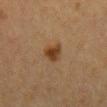Findings:
– biopsy status · no biopsy performed (imaged during a skin exam)
– image · ~15 mm crop, total-body skin-cancer survey
– patient · female, in their mid-50s
– anatomic site · the abdomen
– illumination · cross-polarized
– lesion diameter · ≈2.5 mm